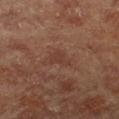Part of a total-body skin-imaging series; this lesion was reviewed on a skin check and was not flagged for biopsy. Cropped from a total-body skin-imaging series; the visible field is about 15 mm. The lesion-visualizer software estimated an average lesion color of about L≈34 a*≈19 b*≈23 (CIELAB), about 5 CIELAB-L* units darker than the surrounding skin, and a normalized lesion–skin contrast near 4.5. About 2.5 mm across. A female patient aged approximately 80. On the chest.